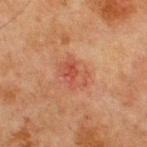Notes:
* workup · no biopsy performed (imaged during a skin exam)
* image · ~15 mm crop, total-body skin-cancer survey
* anatomic site · the chest
* automated metrics · a footprint of about 7 mm², an eccentricity of roughly 0.75, and two-axis asymmetry of about 0.3; a nevus-likeness score of about 0/100
* lighting · cross-polarized illumination
* lesion size · ~3.5 mm (longest diameter)
* patient · male, about 65 years old